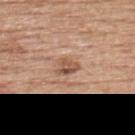follow-up: no biopsy performed (imaged during a skin exam) | body site: the upper back | illumination: white-light illumination | patient: male, aged 58–62 | automated metrics: an area of roughly 3.5 mm² and a shape-asymmetry score of about 0.4 (0 = symmetric); a lesion-to-skin contrast of about 7.5 (normalized; higher = more distinct); an automated nevus-likeness rating near 10 out of 100 and lesion-presence confidence of about 100/100 | image source: total-body-photography crop, ~15 mm field of view | diameter: ~2.5 mm (longest diameter).